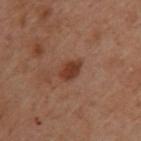{"biopsy_status": "not biopsied; imaged during a skin examination", "automated_metrics": {"border_irregularity_0_10": 1.5, "color_variation_0_10": 1.5, "peripheral_color_asymmetry": 0.5, "nevus_likeness_0_100": 85, "lesion_detection_confidence_0_100": 100}, "lesion_size": {"long_diameter_mm_approx": 2.5}, "patient": {"sex": "female", "age_approx": 55}, "site": "upper back", "image": {"source": "total-body photography crop", "field_of_view_mm": 15}}